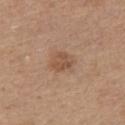Q: Is there a histopathology result?
A: total-body-photography surveillance lesion; no biopsy
Q: How large is the lesion?
A: ≈2.5 mm
Q: What kind of image is this?
A: 15 mm crop, total-body photography
Q: Who is the patient?
A: male, aged 63–67
Q: What did automated image analysis measure?
A: a footprint of about 4.5 mm², a shape eccentricity near 0.5, and a symmetry-axis asymmetry near 0.35; an average lesion color of about L≈51 a*≈19 b*≈31 (CIELAB), roughly 9 lightness units darker than nearby skin, and a normalized border contrast of about 6.5; border irregularity of about 3 on a 0–10 scale and a within-lesion color-variation index near 2/10; an automated nevus-likeness rating near 55 out of 100 and a lesion-detection confidence of about 100/100
Q: What is the anatomic site?
A: the chest